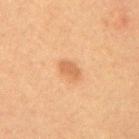notes: total-body-photography surveillance lesion; no biopsy | size: about 2.5 mm | image: ~15 mm crop, total-body skin-cancer survey | patient: female, about 50 years old | automated lesion analysis: a within-lesion color-variation index near 1.5/10 and radial color variation of about 0.5 | location: the upper back.